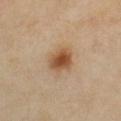Q: Was a biopsy performed?
A: imaged on a skin check; not biopsied
Q: What are the patient's age and sex?
A: female, in their 40s
Q: Automated lesion metrics?
A: a shape eccentricity near 0.55 and a symmetry-axis asymmetry near 0.2; a lesion color around L≈53 a*≈19 b*≈35 in CIELAB, a lesion–skin lightness drop of about 12, and a lesion-to-skin contrast of about 9 (normalized; higher = more distinct)
Q: What is the imaging modality?
A: ~15 mm crop, total-body skin-cancer survey
Q: What is the anatomic site?
A: the chest